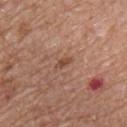Background: A 15 mm crop from a total-body photograph taken for skin-cancer surveillance. Captured under white-light illumination. Automated tile analysis of the lesion measured a lesion color around L≈48 a*≈21 b*≈30 in CIELAB. It also reported a border-irregularity index near 3/10, a color-variation rating of about 0/10, and radial color variation of about 0. The software also gave a nevus-likeness score of about 10/100 and a lesion-detection confidence of about 100/100. The lesion's longest dimension is about 2.5 mm. On the chest. The patient is a male in their mid-50s.